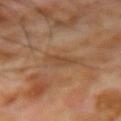follow-up: imaged on a skin check; not biopsied | image source: total-body-photography crop, ~15 mm field of view | body site: the arm | tile lighting: cross-polarized | subject: male, roughly 70 years of age | automated metrics: an area of roughly 3 mm² and a symmetry-axis asymmetry near 0.35; an average lesion color of about L≈44 a*≈19 b*≈32 (CIELAB), about 6 CIELAB-L* units darker than the surrounding skin, and a lesion-to-skin contrast of about 5.5 (normalized; higher = more distinct); a border-irregularity index near 4/10, a color-variation rating of about 0.5/10, and radial color variation of about 0; a nevus-likeness score of about 0/100 and lesion-presence confidence of about 90/100 | lesion size: ≈3 mm.